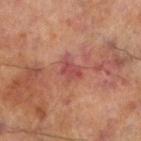| feature | finding |
|---|---|
| biopsy status | no biopsy performed (imaged during a skin exam) |
| automated metrics | an average lesion color of about L≈39 a*≈25 b*≈22 (CIELAB), a lesion–skin lightness drop of about 7, and a normalized lesion–skin contrast near 6.5; a border-irregularity rating of about 4/10 and radial color variation of about 0.5; a nevus-likeness score of about 0/100 and a detector confidence of about 100 out of 100 that the crop contains a lesion |
| tile lighting | cross-polarized |
| image source | 15 mm crop, total-body photography |
| site | the left lower leg |
| size | about 3 mm |
| patient | male, aged approximately 70 |